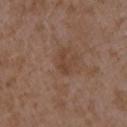biopsy status — imaged on a skin check; not biopsied
lesion size — ≈2.5 mm
acquisition — ~15 mm crop, total-body skin-cancer survey
location — the left forearm
subject — female, in their mid-30s
tile lighting — white-light